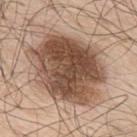{"biopsy_status": "not biopsied; imaged during a skin examination", "site": "upper back", "patient": {"sex": "male", "age_approx": 70}, "image": {"source": "total-body photography crop", "field_of_view_mm": 15}}